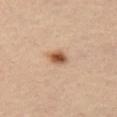<tbp_lesion>
<biopsy_status>not biopsied; imaged during a skin examination</biopsy_status>
<image>
  <source>total-body photography crop</source>
  <field_of_view_mm>15</field_of_view_mm>
</image>
<lighting>cross-polarized</lighting>
<site>left thigh</site>
<patient>
  <sex>male</sex>
  <age_approx>65</age_approx>
</patient>
</tbp_lesion>